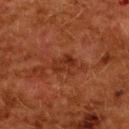Clinical impression: Imaged during a routine full-body skin examination; the lesion was not biopsied and no histopathology is available. Context: A close-up tile cropped from a whole-body skin photograph, about 15 mm across. The lesion-visualizer software estimated a lesion color around L≈26 a*≈24 b*≈28 in CIELAB, roughly 6 lightness units darker than nearby skin, and a normalized border contrast of about 6.5. On the back. A female subject aged approximately 50. Measured at roughly 3 mm in maximum diameter.